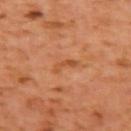Q: Was this lesion biopsied?
A: total-body-photography surveillance lesion; no biopsy
Q: Patient demographics?
A: male, about 60 years old
Q: How was this image acquired?
A: 15 mm crop, total-body photography
Q: Where on the body is the lesion?
A: the left upper arm
Q: What is the lesion's diameter?
A: ~3 mm (longest diameter)
Q: How was the tile lit?
A: cross-polarized
Q: What did automated image analysis measure?
A: an area of roughly 3 mm², an eccentricity of roughly 0.9, and a symmetry-axis asymmetry near 0.5; about 7 CIELAB-L* units darker than the surrounding skin; a within-lesion color-variation index near 0/10 and radial color variation of about 0; an automated nevus-likeness rating near 0 out of 100 and lesion-presence confidence of about 100/100